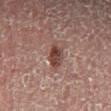follow-up = catalogued during a skin exam; not biopsied
image = ~15 mm tile from a whole-body skin photo
illumination = cross-polarized
subject = male, roughly 50 years of age
lesion size = ≈3 mm
location = the left lower leg